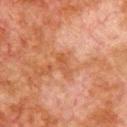The lesion was photographed on a routine skin check and not biopsied; there is no pathology result. This image is a 15 mm lesion crop taken from a total-body photograph. A male subject about 80 years old. The lesion is on the chest. Captured under cross-polarized illumination. About 3 mm across.